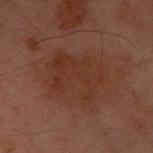Case summary:
* follow-up — total-body-photography surveillance lesion; no biopsy
* patient — female, in their 60s
* image — ~15 mm crop, total-body skin-cancer survey
* lesion size — ~6.5 mm (longest diameter)
* site — the front of the torso